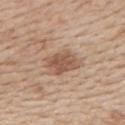- follow-up · imaged on a skin check; not biopsied
- anatomic site · the upper back
- lesion size · about 4 mm
- patient · male, roughly 60 years of age
- automated lesion analysis · roughly 11 lightness units darker than nearby skin and a lesion-to-skin contrast of about 7.5 (normalized; higher = more distinct)
- lighting · white-light illumination
- image · 15 mm crop, total-body photography